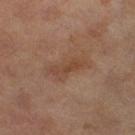Assessment:
No biopsy was performed on this lesion — it was imaged during a full skin examination and was not determined to be concerning.
Image and clinical context:
A female subject, in their 60s. The lesion's longest dimension is about 4.5 mm. A 15 mm close-up tile from a total-body photography series done for melanoma screening. An algorithmic analysis of the crop reported a mean CIELAB color near L≈38 a*≈16 b*≈26 and a normalized border contrast of about 5.5. From the leg.